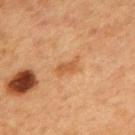{"biopsy_status": "not biopsied; imaged during a skin examination", "patient": {"sex": "female", "age_approx": 40}, "lighting": "cross-polarized", "site": "mid back", "image": {"source": "total-body photography crop", "field_of_view_mm": 15}}